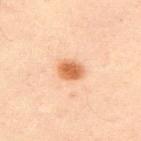<case>
  <biopsy_status>not biopsied; imaged during a skin examination</biopsy_status>
  <site>upper back</site>
  <patient>
    <sex>male</sex>
    <age_approx>50</age_approx>
  </patient>
  <image>
    <source>total-body photography crop</source>
    <field_of_view_mm>15</field_of_view_mm>
  </image>
</case>